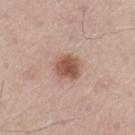An algorithmic analysis of the crop reported a color-variation rating of about 3.5/10. The lesion is located on the left thigh. Cropped from a total-body skin-imaging series; the visible field is about 15 mm. A male patient, approximately 75 years of age.